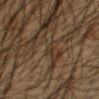This lesion was catalogued during total-body skin photography and was not selected for biopsy. Captured under cross-polarized illumination. The total-body-photography lesion software estimated a lesion color around L≈33 a*≈12 b*≈25 in CIELAB and a lesion–skin lightness drop of about 4. It also reported a border-irregularity rating of about 3.5/10 and a within-lesion color-variation index near 0/10. It also reported an automated nevus-likeness rating near 0 out of 100 and a lesion-detection confidence of about 0/100. A roughly 15 mm field-of-view crop from a total-body skin photograph. A male patient aged 43–47. Located on the left forearm. The lesion's longest dimension is about 1.5 mm.